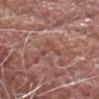Notes:
– follow-up — catalogued during a skin exam; not biopsied
– body site — the head or neck
– automated lesion analysis — an average lesion color of about L≈48 a*≈23 b*≈25 (CIELAB) and a normalized lesion–skin contrast near 5; a border-irregularity index near 8/10, a color-variation rating of about 1/10, and peripheral color asymmetry of about 0.5; a nevus-likeness score of about 0/100 and lesion-presence confidence of about 85/100
– subject — male, aged 68–72
– lesion diameter — ~3 mm (longest diameter)
– tile lighting — white-light
– imaging modality — total-body-photography crop, ~15 mm field of view